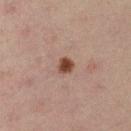Captured during whole-body skin photography for melanoma surveillance; the lesion was not biopsied. From the left upper arm. A roughly 15 mm field-of-view crop from a total-body skin photograph. A male patient aged 28–32. About 2 mm across. This is a cross-polarized tile.